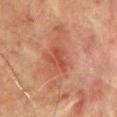Impression: This lesion was catalogued during total-body skin photography and was not selected for biopsy. Background: A male patient about 65 years old. A lesion tile, about 15 mm wide, cut from a 3D total-body photograph. Measured at roughly 3 mm in maximum diameter. This is a cross-polarized tile. From the chest.